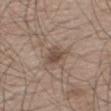Clinical impression: No biopsy was performed on this lesion — it was imaged during a full skin examination and was not determined to be concerning. Background: Imaged with white-light lighting. Measured at roughly 3.5 mm in maximum diameter. The lesion is located on the right thigh. Cropped from a total-body skin-imaging series; the visible field is about 15 mm. An algorithmic analysis of the crop reported a lesion area of about 5.5 mm², an eccentricity of roughly 0.7, and two-axis asymmetry of about 0.35. The analysis additionally found border irregularity of about 3 on a 0–10 scale, internal color variation of about 3 on a 0–10 scale, and peripheral color asymmetry of about 1. The analysis additionally found a nevus-likeness score of about 50/100 and lesion-presence confidence of about 100/100. The subject is a male about 60 years old.